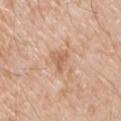{
  "biopsy_status": "not biopsied; imaged during a skin examination",
  "image": {
    "source": "total-body photography crop",
    "field_of_view_mm": 15
  },
  "site": "chest",
  "automated_metrics": {
    "vs_skin_darker_L": 9.0,
    "color_variation_0_10": 1.5,
    "peripheral_color_asymmetry": 0.5,
    "nevus_likeness_0_100": 0,
    "lesion_detection_confidence_0_100": 100
  },
  "lighting": "white-light",
  "patient": {
    "sex": "male",
    "age_approx": 65
  },
  "lesion_size": {
    "long_diameter_mm_approx": 2.5
  }
}